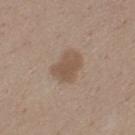No biopsy was performed on this lesion — it was imaged during a full skin examination and was not determined to be concerning.
The total-body-photography lesion software estimated an outline eccentricity of about 0.7 (0 = round, 1 = elongated).
A female subject roughly 35 years of age.
A close-up tile cropped from a whole-body skin photograph, about 15 mm across.
Measured at roughly 3.5 mm in maximum diameter.
Located on the leg.